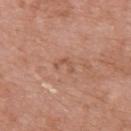follow-up = no biopsy performed (imaged during a skin exam) | patient = female, about 70 years old | image = total-body-photography crop, ~15 mm field of view | diameter = about 2.5 mm | anatomic site = the upper back | tile lighting = white-light illumination | automated lesion analysis = border irregularity of about 8 on a 0–10 scale, a color-variation rating of about 0/10, and a peripheral color-asymmetry measure near 0.